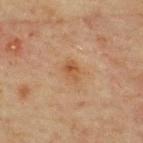biopsy_status: not biopsied; imaged during a skin examination
lesion_size:
  long_diameter_mm_approx: 2.5
lighting: cross-polarized
site: upper back
patient:
  sex: female
  age_approx: 40
image:
  source: total-body photography crop
  field_of_view_mm: 15